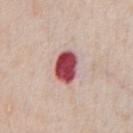{"biopsy_status": "not biopsied; imaged during a skin examination", "patient": {"sex": "male", "age_approx": 60}, "site": "chest", "image": {"source": "total-body photography crop", "field_of_view_mm": 15}}